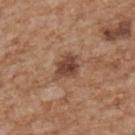Imaged during a routine full-body skin examination; the lesion was not biopsied and no histopathology is available.
The tile uses white-light illumination.
From the upper back.
Approximately 3.5 mm at its widest.
A 15 mm close-up tile from a total-body photography series done for melanoma screening.
The patient is a male roughly 65 years of age.
An algorithmic analysis of the crop reported a lesion area of about 7.5 mm², an eccentricity of roughly 0.65, and a symmetry-axis asymmetry near 0.3. The software also gave an average lesion color of about L≈44 a*≈21 b*≈28 (CIELAB). The software also gave a border-irregularity rating of about 3/10, internal color variation of about 3.5 on a 0–10 scale, and radial color variation of about 1. It also reported a nevus-likeness score of about 30/100 and a detector confidence of about 100 out of 100 that the crop contains a lesion.